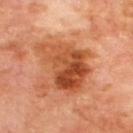<record>
  <biopsy_status>not biopsied; imaged during a skin examination</biopsy_status>
  <site>back</site>
  <lighting>cross-polarized</lighting>
  <lesion_size>
    <long_diameter_mm_approx>7.0</long_diameter_mm_approx>
  </lesion_size>
  <image>
    <source>total-body photography crop</source>
    <field_of_view_mm>15</field_of_view_mm>
  </image>
  <patient>
    <sex>male</sex>
    <age_approx>70</age_approx>
  </patient>
</record>